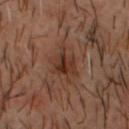<record>
  <biopsy_status>not biopsied; imaged during a skin examination</biopsy_status>
  <image>
    <source>total-body photography crop</source>
    <field_of_view_mm>15</field_of_view_mm>
  </image>
  <patient>
    <sex>male</sex>
    <age_approx>50</age_approx>
  </patient>
  <site>head or neck</site>
</record>